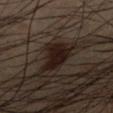Part of a total-body skin-imaging series; this lesion was reviewed on a skin check and was not flagged for biopsy. The lesion-visualizer software estimated a mean CIELAB color near L≈11 a*≈9 b*≈11, a lesion–skin lightness drop of about 8, and a normalized lesion–skin contrast near 13.5. The analysis additionally found an automated nevus-likeness rating near 100 out of 100 and lesion-presence confidence of about 100/100. A male subject about 50 years old. Measured at roughly 4.5 mm in maximum diameter. A roughly 15 mm field-of-view crop from a total-body skin photograph. Captured under cross-polarized illumination. The lesion is on the right lower leg.